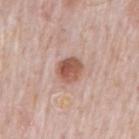This lesion was catalogued during total-body skin photography and was not selected for biopsy.
A 15 mm close-up extracted from a 3D total-body photography capture.
The lesion is on the mid back.
A male patient, roughly 80 years of age.